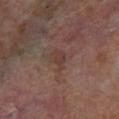Assessment: Recorded during total-body skin imaging; not selected for excision or biopsy. Clinical summary: A lesion tile, about 15 mm wide, cut from a 3D total-body photograph. Captured under cross-polarized illumination. The lesion is located on the right lower leg. About 2.5 mm across. A male patient, roughly 70 years of age.